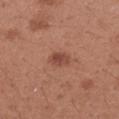{"biopsy_status": "not biopsied; imaged during a skin examination", "lighting": "white-light", "automated_metrics": {"area_mm2_approx": 4.0, "eccentricity": 0.8, "shape_asymmetry": 0.25}, "lesion_size": {"long_diameter_mm_approx": 3.0}, "image": {"source": "total-body photography crop", "field_of_view_mm": 15}, "patient": {"sex": "female", "age_approx": 30}, "site": "right forearm"}